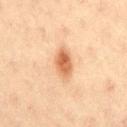Part of a total-body skin-imaging series; this lesion was reviewed on a skin check and was not flagged for biopsy. A 15 mm close-up tile from a total-body photography series done for melanoma screening. The tile uses cross-polarized illumination. A female subject, aged around 40. Located on the lower back. About 4 mm across.